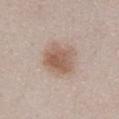Case summary:
* notes · total-body-photography surveillance lesion; no biopsy
* image · total-body-photography crop, ~15 mm field of view
* patient · female, aged 48–52
* location · the abdomen
* tile lighting · white-light illumination
* image-analysis metrics · a lesion area of about 14 mm², a shape eccentricity near 0.5, and two-axis asymmetry of about 0.2; an average lesion color of about L≈58 a*≈17 b*≈26 (CIELAB), about 10 CIELAB-L* units darker than the surrounding skin, and a normalized lesion–skin contrast near 7.5; an automated nevus-likeness rating near 95 out of 100 and lesion-presence confidence of about 100/100